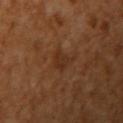Q: How large is the lesion?
A: about 2.5 mm
Q: How was the tile lit?
A: cross-polarized illumination
Q: What kind of image is this?
A: 15 mm crop, total-body photography
Q: Patient demographics?
A: female, roughly 55 years of age
Q: Automated lesion metrics?
A: a detector confidence of about 100 out of 100 that the crop contains a lesion
Q: What is the anatomic site?
A: the right upper arm